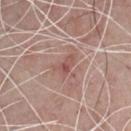  biopsy_status: not biopsied; imaged during a skin examination
  site: front of the torso
  patient:
    sex: male
    age_approx: 70
  image:
    source: total-body photography crop
    field_of_view_mm: 15